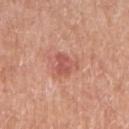Notes:
* follow-up — no biopsy performed (imaged during a skin exam)
* lesion size — about 3 mm
* body site — the right upper arm
* TBP lesion metrics — a lesion area of about 5 mm² and an eccentricity of roughly 0.5; a lesion color around L≈55 a*≈29 b*≈29 in CIELAB and about 9 CIELAB-L* units darker than the surrounding skin; border irregularity of about 4 on a 0–10 scale, a color-variation rating of about 3/10, and a peripheral color-asymmetry measure near 1
* image — ~15 mm crop, total-body skin-cancer survey
* tile lighting — white-light
* subject — female, aged 73–77